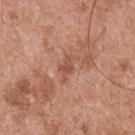Impression: The lesion was tiled from a total-body skin photograph and was not biopsied. Clinical summary: Imaged with white-light lighting. Located on the upper back. A male patient, aged 53–57. A lesion tile, about 15 mm wide, cut from a 3D total-body photograph.